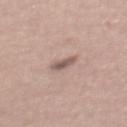Q: Illumination type?
A: white-light illumination
Q: What are the patient's age and sex?
A: male, aged 38 to 42
Q: Lesion location?
A: the mid back
Q: What is the lesion's diameter?
A: ~2.5 mm (longest diameter)
Q: How was this image acquired?
A: ~15 mm crop, total-body skin-cancer survey
Q: Automated lesion metrics?
A: lesion-presence confidence of about 100/100